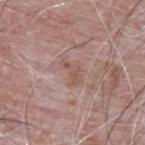This lesion was catalogued during total-body skin photography and was not selected for biopsy. A male patient in their mid-60s. The lesion is located on the upper back. Longest diameter approximately 3 mm. Cropped from a total-body skin-imaging series; the visible field is about 15 mm. Imaged with white-light lighting.